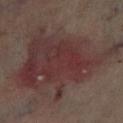Impression: This lesion was catalogued during total-body skin photography and was not selected for biopsy. Context: The lesion's longest dimension is about 14 mm. On the leg. A male subject, approximately 70 years of age. A close-up tile cropped from a whole-body skin photograph, about 15 mm across.